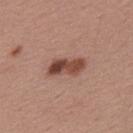This lesion was catalogued during total-body skin photography and was not selected for biopsy. A lesion tile, about 15 mm wide, cut from a 3D total-body photograph. From the back. The patient is a male aged 53–57. About 4 mm across.